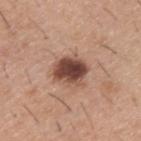Captured during whole-body skin photography for melanoma surveillance; the lesion was not biopsied. The total-body-photography lesion software estimated a footprint of about 10 mm², an eccentricity of roughly 0.5, and a shape-asymmetry score of about 0.2 (0 = symmetric). It also reported border irregularity of about 2 on a 0–10 scale, a color-variation rating of about 6.5/10, and peripheral color asymmetry of about 2. It also reported an automated nevus-likeness rating near 80 out of 100 and a detector confidence of about 100 out of 100 that the crop contains a lesion. Captured under white-light illumination. A male subject, aged approximately 40. A lesion tile, about 15 mm wide, cut from a 3D total-body photograph. Located on the upper back.